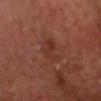Recorded during total-body skin imaging; not selected for excision or biopsy. Longest diameter approximately 4 mm. On the head or neck. A lesion tile, about 15 mm wide, cut from a 3D total-body photograph. This is a cross-polarized tile. A male subject, approximately 65 years of age. An algorithmic analysis of the crop reported a lesion color around L≈34 a*≈24 b*≈28 in CIELAB, roughly 6 lightness units darker than nearby skin, and a lesion-to-skin contrast of about 5.5 (normalized; higher = more distinct). It also reported a border-irregularity index near 5.5/10, internal color variation of about 2.5 on a 0–10 scale, and radial color variation of about 1.